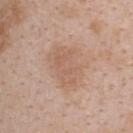This lesion was catalogued during total-body skin photography and was not selected for biopsy. The tile uses white-light illumination. The lesion's longest dimension is about 5.5 mm. A female subject, aged around 45. The lesion is located on the upper back. Cropped from a total-body skin-imaging series; the visible field is about 15 mm. An algorithmic analysis of the crop reported a lesion area of about 18 mm², an outline eccentricity of about 0.6 (0 = round, 1 = elongated), and two-axis asymmetry of about 0.25. And it measured a border-irregularity rating of about 2.5/10, a color-variation rating of about 3/10, and a peripheral color-asymmetry measure near 1. And it measured a lesion-detection confidence of about 100/100.